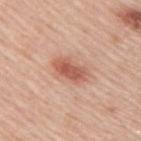Findings:
- biopsy status — catalogued during a skin exam; not biopsied
- anatomic site — the upper back
- lighting — white-light illumination
- subject — male, about 45 years old
- image — ~15 mm crop, total-body skin-cancer survey
- lesion diameter — ~4 mm (longest diameter)